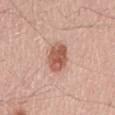Q: Illumination type?
A: white-light
Q: What is the anatomic site?
A: the mid back
Q: What is the lesion's diameter?
A: ~3.5 mm (longest diameter)
Q: What kind of image is this?
A: ~15 mm crop, total-body skin-cancer survey
Q: What are the patient's age and sex?
A: male, approximately 60 years of age
Q: What did automated image analysis measure?
A: a lesion color around L≈57 a*≈24 b*≈29 in CIELAB, about 13 CIELAB-L* units darker than the surrounding skin, and a lesion-to-skin contrast of about 9 (normalized; higher = more distinct); a nevus-likeness score of about 95/100 and a lesion-detection confidence of about 100/100